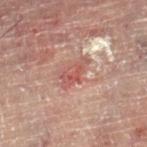Q: Was a biopsy performed?
A: no biopsy performed (imaged during a skin exam)
Q: Where on the body is the lesion?
A: the right lower leg
Q: What is the imaging modality?
A: total-body-photography crop, ~15 mm field of view
Q: Lesion size?
A: ~4 mm (longest diameter)
Q: Patient demographics?
A: male, approximately 60 years of age
Q: What did automated image analysis measure?
A: a footprint of about 5 mm², an eccentricity of roughly 0.9, and a symmetry-axis asymmetry near 0.4
Q: How was the tile lit?
A: cross-polarized